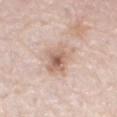Assessment: The lesion was tiled from a total-body skin photograph and was not biopsied. Acquisition and patient details: A 15 mm close-up extracted from a 3D total-body photography capture. The subject is a male roughly 80 years of age. On the left thigh.